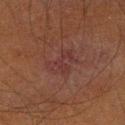Captured during whole-body skin photography for melanoma surveillance; the lesion was not biopsied.
Cropped from a total-body skin-imaging series; the visible field is about 15 mm.
This is a cross-polarized tile.
The lesion is on the right lower leg.
A male subject, aged approximately 40.